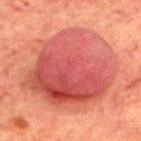No biopsy was performed on this lesion — it was imaged during a full skin examination and was not determined to be concerning.
The tile uses cross-polarized illumination.
From the back.
The lesion's longest dimension is about 11 mm.
A 15 mm close-up tile from a total-body photography series done for melanoma screening.
The total-body-photography lesion software estimated an average lesion color of about L≈54 a*≈36 b*≈31 (CIELAB), about 12 CIELAB-L* units darker than the surrounding skin, and a normalized lesion–skin contrast near 8.5. The analysis additionally found border irregularity of about 2 on a 0–10 scale, a within-lesion color-variation index near 9.5/10, and radial color variation of about 3. And it measured an automated nevus-likeness rating near 20 out of 100 and lesion-presence confidence of about 100/100.
A male subject approximately 70 years of age.